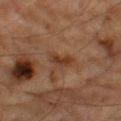The lesion was photographed on a routine skin check and not biopsied; there is no pathology result. A male patient, aged around 85 or older. From the left thigh. Measured at roughly 3.5 mm in maximum diameter. Automated image analysis of the tile measured a within-lesion color-variation index near 2.5/10 and a peripheral color-asymmetry measure near 1. The software also gave an automated nevus-likeness rating near 0 out of 100 and a detector confidence of about 100 out of 100 that the crop contains a lesion. This image is a 15 mm lesion crop taken from a total-body photograph.